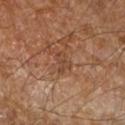workup: imaged on a skin check; not biopsied | TBP lesion metrics: two-axis asymmetry of about 0.5; a color-variation rating of about 1.5/10 and a peripheral color-asymmetry measure near 0.5; a nevus-likeness score of about 0/100 and a detector confidence of about 95 out of 100 that the crop contains a lesion | lesion size: ~2.5 mm (longest diameter) | image: ~15 mm crop, total-body skin-cancer survey | patient: male, aged 83 or over | illumination: cross-polarized illumination | anatomic site: the right forearm.